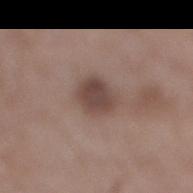follow-up = imaged on a skin check; not biopsied
anatomic site = the right lower leg
automated lesion analysis = an area of roughly 8 mm², a shape eccentricity near 0.5, and a symmetry-axis asymmetry near 0.2; a mean CIELAB color near L≈45 a*≈16 b*≈21, a lesion–skin lightness drop of about 11, and a normalized border contrast of about 8.5; a within-lesion color-variation index near 2.5/10 and radial color variation of about 1
image = ~15 mm tile from a whole-body skin photo
illumination = white-light illumination
patient = male, roughly 50 years of age
diameter = ≈3.5 mm